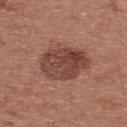Assessment: This lesion was catalogued during total-body skin photography and was not selected for biopsy. Image and clinical context: The lesion-visualizer software estimated a footprint of about 19 mm², a shape eccentricity near 0.65, and a shape-asymmetry score of about 0.15 (0 = symmetric). And it measured a border-irregularity index near 2/10. It also reported a lesion-detection confidence of about 100/100. The subject is a female aged 38–42. This is a white-light tile. The lesion is on the upper back. About 6 mm across. Cropped from a total-body skin-imaging series; the visible field is about 15 mm.